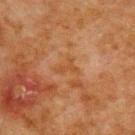Q: Was a biopsy performed?
A: no biopsy performed (imaged during a skin exam)
Q: What kind of image is this?
A: 15 mm crop, total-body photography
Q: What is the anatomic site?
A: the upper back
Q: How large is the lesion?
A: about 3 mm
Q: How was the tile lit?
A: cross-polarized illumination
Q: What are the patient's age and sex?
A: male, aged 78 to 82